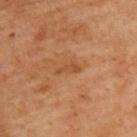Impression:
The lesion was photographed on a routine skin check and not biopsied; there is no pathology result.
Context:
The subject is a male aged 63–67. Automated image analysis of the tile measured an eccentricity of roughly 0.95 and a shape-asymmetry score of about 0.4 (0 = symmetric). The software also gave an average lesion color of about L≈48 a*≈24 b*≈38 (CIELAB) and roughly 7 lightness units darker than nearby skin. From the upper back. A close-up tile cropped from a whole-body skin photograph, about 15 mm across. This is a cross-polarized tile.